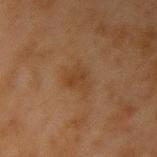Q: Was this lesion biopsied?
A: catalogued during a skin exam; not biopsied